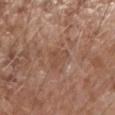Impression: The lesion was tiled from a total-body skin photograph and was not biopsied. Image and clinical context: Approximately 3 mm at its widest. Located on the left forearm. Automated tile analysis of the lesion measured about 6 CIELAB-L* units darker than the surrounding skin and a normalized lesion–skin contrast near 5. The software also gave a border-irregularity rating of about 6.5/10 and a within-lesion color-variation index near 0.5/10. The analysis additionally found an automated nevus-likeness rating near 0 out of 100 and a lesion-detection confidence of about 95/100. This is a white-light tile. A roughly 15 mm field-of-view crop from a total-body skin photograph. The subject is a male approximately 75 years of age.